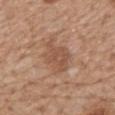notes: total-body-photography surveillance lesion; no biopsy | site: the mid back | subject: male, about 70 years old | image source: 15 mm crop, total-body photography.